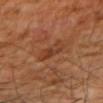On the right upper arm. A male patient aged approximately 60. A region of skin cropped from a whole-body photographic capture, roughly 15 mm wide.